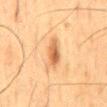Recorded during total-body skin imaging; not selected for excision or biopsy.
Located on the mid back.
The total-body-photography lesion software estimated an area of roughly 5.5 mm², a shape eccentricity near 0.9, and two-axis asymmetry of about 0.3. The analysis additionally found about 11 CIELAB-L* units darker than the surrounding skin and a lesion-to-skin contrast of about 8 (normalized; higher = more distinct). And it measured an automated nevus-likeness rating near 90 out of 100 and a lesion-detection confidence of about 100/100.
The subject is a male approximately 60 years of age.
A 15 mm crop from a total-body photograph taken for skin-cancer surveillance.
The tile uses cross-polarized illumination.